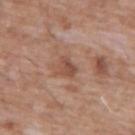Q: Is there a histopathology result?
A: total-body-photography surveillance lesion; no biopsy
Q: Lesion location?
A: the chest
Q: What are the patient's age and sex?
A: male, roughly 60 years of age
Q: What lighting was used for the tile?
A: white-light illumination
Q: How was this image acquired?
A: ~15 mm tile from a whole-body skin photo